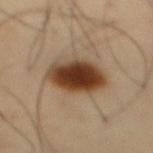workup=imaged on a skin check; not biopsied | location=the front of the torso | lighting=cross-polarized illumination | image=~15 mm tile from a whole-body skin photo | subject=male, about 55 years old.